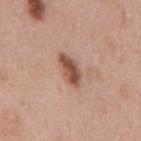The lesion was photographed on a routine skin check and not biopsied; there is no pathology result.
From the back.
A male patient, roughly 60 years of age.
A roughly 15 mm field-of-view crop from a total-body skin photograph.